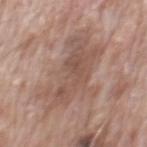No biopsy was performed on this lesion — it was imaged during a full skin examination and was not determined to be concerning. The recorded lesion diameter is about 9.5 mm. From the back. A region of skin cropped from a whole-body photographic capture, roughly 15 mm wide. The subject is a male about 70 years old.